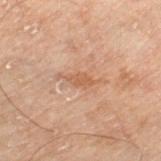biopsy_status: not biopsied; imaged during a skin examination
site: right lower leg
lighting: cross-polarized
patient:
  sex: male
  age_approx: 70
image:
  source: total-body photography crop
  field_of_view_mm: 15
lesion_size:
  long_diameter_mm_approx: 3.0